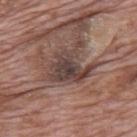This lesion was catalogued during total-body skin photography and was not selected for biopsy.
From the mid back.
A male subject aged 68–72.
A lesion tile, about 15 mm wide, cut from a 3D total-body photograph.